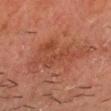biopsy status — catalogued during a skin exam; not biopsied
imaging modality — ~15 mm crop, total-body skin-cancer survey
patient — male, in their mid-40s
body site — the head or neck
automated lesion analysis — an area of roughly 20 mm², an eccentricity of roughly 0.9, and a symmetry-axis asymmetry near 0.5; a lesion color around L≈37 a*≈23 b*≈27 in CIELAB, a lesion–skin lightness drop of about 6, and a normalized lesion–skin contrast near 5.5; a lesion-detection confidence of about 100/100
illumination — cross-polarized illumination
diameter — ~8 mm (longest diameter)